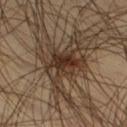Clinical impression:
Recorded during total-body skin imaging; not selected for excision or biopsy.
Acquisition and patient details:
A lesion tile, about 15 mm wide, cut from a 3D total-body photograph. This is a cross-polarized tile. From the right thigh. A male patient, aged 43 to 47. About 9.5 mm across.